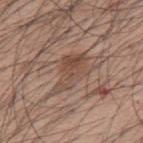This lesion was catalogued during total-body skin photography and was not selected for biopsy. The lesion-visualizer software estimated a lesion area of about 11 mm² and a symmetry-axis asymmetry near 0.35. It also reported roughly 8 lightness units darker than nearby skin and a normalized border contrast of about 6. The lesion is on the back. The subject is a male aged approximately 60. A region of skin cropped from a whole-body photographic capture, roughly 15 mm wide. The tile uses white-light illumination.